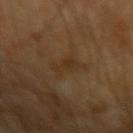<lesion>
<image>
  <source>total-body photography crop</source>
  <field_of_view_mm>15</field_of_view_mm>
</image>
<patient>
  <sex>male</sex>
  <age_approx>65</age_approx>
</patient>
</lesion>